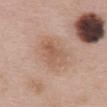| key | value |
|---|---|
| image source | 15 mm crop, total-body photography |
| subject | female, aged approximately 60 |
| body site | the mid back |